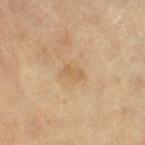| key | value |
|---|---|
| workup | total-body-photography surveillance lesion; no biopsy |
| site | the right thigh |
| automated metrics | a shape eccentricity near 0.85 and a shape-asymmetry score of about 0.3 (0 = symmetric); a mean CIELAB color near L≈51 a*≈15 b*≈33 and a normalized lesion–skin contrast near 5 |
| image | ~15 mm tile from a whole-body skin photo |
| lighting | cross-polarized |
| size | ~2.5 mm (longest diameter) |
| patient | female, about 80 years old |